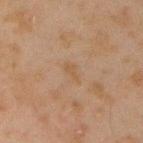Q: Is there a histopathology result?
A: catalogued during a skin exam; not biopsied
Q: Where on the body is the lesion?
A: the right upper arm
Q: What kind of image is this?
A: ~15 mm crop, total-body skin-cancer survey
Q: What is the lesion's diameter?
A: about 2.5 mm
Q: Who is the patient?
A: male, aged 43 to 47